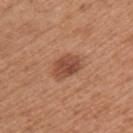<case>
<biopsy_status>not biopsied; imaged during a skin examination</biopsy_status>
<patient>
  <sex>female</sex>
  <age_approx>40</age_approx>
</patient>
<site>upper back</site>
<automated_metrics>
  <area_mm2_approx>7.5</area_mm2_approx>
  <eccentricity>0.65</eccentricity>
  <shape_asymmetry>0.2</shape_asymmetry>
</automated_metrics>
<image>
  <source>total-body photography crop</source>
  <field_of_view_mm>15</field_of_view_mm>
</image>
<lesion_size>
  <long_diameter_mm_approx>3.5</long_diameter_mm_approx>
</lesion_size>
<lighting>white-light</lighting>
</case>